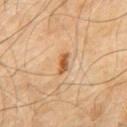• follow-up: total-body-photography surveillance lesion; no biopsy
• anatomic site: the chest
• lesion diameter: ≈2.5 mm
• subject: male, aged approximately 60
• TBP lesion metrics: a mean CIELAB color near L≈55 a*≈23 b*≈39 and roughly 13 lightness units darker than nearby skin; border irregularity of about 2.5 on a 0–10 scale, a within-lesion color-variation index near 3/10, and radial color variation of about 1
• imaging modality: ~15 mm tile from a whole-body skin photo